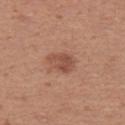The lesion was photographed on a routine skin check and not biopsied; there is no pathology result. This is a white-light tile. The patient is a female aged approximately 40. From the left thigh. An algorithmic analysis of the crop reported an area of roughly 6 mm², an outline eccentricity of about 0.7 (0 = round, 1 = elongated), and two-axis asymmetry of about 0.2. A region of skin cropped from a whole-body photographic capture, roughly 15 mm wide.